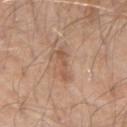workup: total-body-photography surveillance lesion; no biopsy | diameter: ≈3.5 mm | subject: male, aged 58–62 | location: the arm | TBP lesion metrics: a shape eccentricity near 0.95 and two-axis asymmetry of about 0.35 | acquisition: total-body-photography crop, ~15 mm field of view | illumination: white-light.